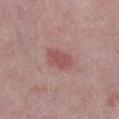Background: This is a white-light tile. The lesion's longest dimension is about 3.5 mm. From the left lower leg. The patient is a male roughly 75 years of age. A roughly 15 mm field-of-view crop from a total-body skin photograph. The lesion-visualizer software estimated a border-irregularity index near 2.5/10, a within-lesion color-variation index near 1.5/10, and peripheral color asymmetry of about 0.5. It also reported a nevus-likeness score of about 65/100.